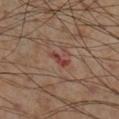Clinical impression:
Captured during whole-body skin photography for melanoma surveillance; the lesion was not biopsied.
Clinical summary:
The total-body-photography lesion software estimated an outline eccentricity of about 0.95 (0 = round, 1 = elongated) and two-axis asymmetry of about 0.45. The software also gave a border-irregularity index near 5/10. A lesion tile, about 15 mm wide, cut from a 3D total-body photograph. Captured under cross-polarized illumination. A male patient aged 53 to 57. Approximately 2.5 mm at its widest. The lesion is located on the right lower leg.